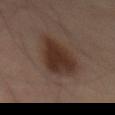Assessment: Imaged during a routine full-body skin examination; the lesion was not biopsied and no histopathology is available. Context: A lesion tile, about 15 mm wide, cut from a 3D total-body photograph. A male subject aged 68 to 72. Located on the front of the torso. About 6 mm across.